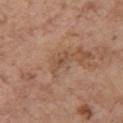Captured during whole-body skin photography for melanoma surveillance; the lesion was not biopsied.
From the left upper arm.
A 15 mm crop from a total-body photograph taken for skin-cancer surveillance.
Automated tile analysis of the lesion measured an automated nevus-likeness rating near 0 out of 100 and a lesion-detection confidence of about 100/100.
A female patient about 65 years old.
About 3 mm across.